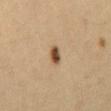{"biopsy_status": "not biopsied; imaged during a skin examination"}